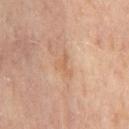{"biopsy_status": "not biopsied; imaged during a skin examination", "site": "leg", "automated_metrics": {"cielab_L": 59, "cielab_a": 20, "cielab_b": 33, "vs_skin_darker_L": 7.0, "vs_skin_contrast_norm": 5.0, "nevus_likeness_0_100": 0, "lesion_detection_confidence_0_100": 100}, "patient": {"sex": "female", "age_approx": 50}, "lesion_size": {"long_diameter_mm_approx": 3.0}, "image": {"source": "total-body photography crop", "field_of_view_mm": 15}}